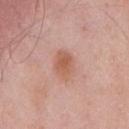Q: Is there a histopathology result?
A: total-body-photography surveillance lesion; no biopsy
Q: How was this image acquired?
A: ~15 mm tile from a whole-body skin photo
Q: How was the tile lit?
A: white-light illumination
Q: Automated lesion metrics?
A: a shape eccentricity near 0.75 and two-axis asymmetry of about 0.2; an average lesion color of about L≈58 a*≈24 b*≈30 (CIELAB) and a normalized lesion–skin contrast near 7; a border-irregularity index near 2/10, a within-lesion color-variation index near 3/10, and a peripheral color-asymmetry measure near 1; a nevus-likeness score of about 75/100 and a detector confidence of about 100 out of 100 that the crop contains a lesion
Q: How large is the lesion?
A: ~3.5 mm (longest diameter)
Q: Patient demographics?
A: male, aged 53 to 57
Q: What is the anatomic site?
A: the chest